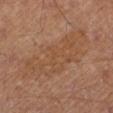Captured during whole-body skin photography for melanoma surveillance; the lesion was not biopsied.
The tile uses cross-polarized illumination.
A male patient aged approximately 60.
Cropped from a total-body skin-imaging series; the visible field is about 15 mm.
The lesion is located on the left lower leg.